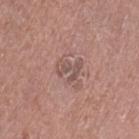No biopsy was performed on this lesion — it was imaged during a full skin examination and was not determined to be concerning. A female subject aged 48–52. On the left thigh. This image is a 15 mm lesion crop taken from a total-body photograph. Imaged with white-light lighting. Longest diameter approximately 3 mm. An algorithmic analysis of the crop reported an eccentricity of roughly 0.65 and a shape-asymmetry score of about 0.5 (0 = symmetric).